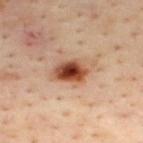No biopsy was performed on this lesion — it was imaged during a full skin examination and was not determined to be concerning. The lesion's longest dimension is about 5 mm. A male subject aged around 45. The tile uses cross-polarized illumination. Cropped from a total-body skin-imaging series; the visible field is about 15 mm. Automated image analysis of the tile measured a lesion area of about 11 mm². The analysis additionally found a lesion color around L≈50 a*≈23 b*≈33 in CIELAB, roughly 16 lightness units darker than nearby skin, and a lesion-to-skin contrast of about 11 (normalized; higher = more distinct). The lesion is on the upper back.